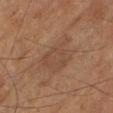biopsy_status: not biopsied; imaged during a skin examination
patient:
  sex: male
  age_approx: 85+
image:
  source: total-body photography crop
  field_of_view_mm: 15
site: leg